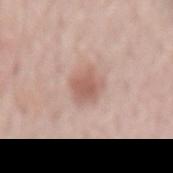The lesion was photographed on a routine skin check and not biopsied; there is no pathology result. Captured under white-light illumination. A lesion tile, about 15 mm wide, cut from a 3D total-body photograph. Longest diameter approximately 3.5 mm. A male patient aged approximately 70. Located on the lower back.